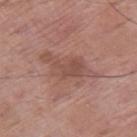A 15 mm crop from a total-body photograph taken for skin-cancer surveillance. A male patient, aged 68 to 72. The lesion's longest dimension is about 6.5 mm. The lesion is located on the right thigh. Captured under white-light illumination.